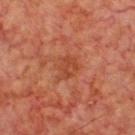| feature | finding |
|---|---|
| follow-up | catalogued during a skin exam; not biopsied |
| patient | male, aged around 65 |
| site | the chest |
| acquisition | 15 mm crop, total-body photography |
| image-analysis metrics | border irregularity of about 3 on a 0–10 scale, a within-lesion color-variation index near 3/10, and peripheral color asymmetry of about 1 |